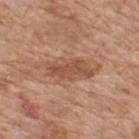Part of a total-body skin-imaging series; this lesion was reviewed on a skin check and was not flagged for biopsy. The tile uses white-light illumination. Measured at roughly 5.5 mm in maximum diameter. A male patient in their 60s. On the back. This image is a 15 mm lesion crop taken from a total-body photograph. The total-body-photography lesion software estimated an area of roughly 10 mm² and an eccentricity of roughly 0.9. The analysis additionally found an average lesion color of about L≈52 a*≈21 b*≈31 (CIELAB), a lesion–skin lightness drop of about 9, and a normalized border contrast of about 6.5. And it measured a classifier nevus-likeness of about 0/100 and a lesion-detection confidence of about 100/100.